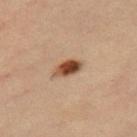Impression:
Recorded during total-body skin imaging; not selected for excision or biopsy.
Clinical summary:
An algorithmic analysis of the crop reported border irregularity of about 2 on a 0–10 scale and a peripheral color-asymmetry measure near 2.5. And it measured a classifier nevus-likeness of about 100/100. The lesion is located on the right thigh. Approximately 3.5 mm at its widest. A close-up tile cropped from a whole-body skin photograph, about 15 mm across. The patient is a female aged around 35.